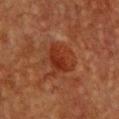Clinical impression:
No biopsy was performed on this lesion — it was imaged during a full skin examination and was not determined to be concerning.
Clinical summary:
The lesion is located on the chest. Cropped from a whole-body photographic skin survey; the tile spans about 15 mm. Longest diameter approximately 4.5 mm. A female patient aged approximately 40.